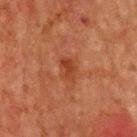biopsy status: imaged on a skin check; not biopsied | illumination: cross-polarized | patient: male, roughly 65 years of age | automated metrics: an area of roughly 3.5 mm², a shape eccentricity near 0.85, and a shape-asymmetry score of about 0.3 (0 = symmetric); an automated nevus-likeness rating near 0 out of 100 and a lesion-detection confidence of about 100/100 | body site: the chest | acquisition: 15 mm crop, total-body photography.